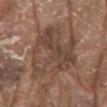Q: Was a biopsy performed?
A: imaged on a skin check; not biopsied
Q: Illumination type?
A: white-light
Q: What is the imaging modality?
A: total-body-photography crop, ~15 mm field of view
Q: Who is the patient?
A: male, roughly 80 years of age
Q: Lesion location?
A: the chest